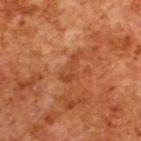The lesion was tiled from a total-body skin photograph and was not biopsied.
The tile uses cross-polarized illumination.
A male patient aged around 80.
Longest diameter approximately 3.5 mm.
The lesion is on the back.
A close-up tile cropped from a whole-body skin photograph, about 15 mm across.